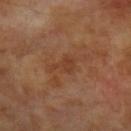Imaged during a routine full-body skin examination; the lesion was not biopsied and no histopathology is available. On the arm. A male subject roughly 70 years of age. This is a cross-polarized tile. The recorded lesion diameter is about 3.5 mm. A region of skin cropped from a whole-body photographic capture, roughly 15 mm wide.